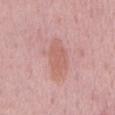<record>
  <biopsy_status>not biopsied; imaged during a skin examination</biopsy_status>
  <patient>
    <sex>male</sex>
    <age_approx>60</age_approx>
  </patient>
  <image>
    <source>total-body photography crop</source>
    <field_of_view_mm>15</field_of_view_mm>
  </image>
  <site>back</site>
  <lesion_size>
    <long_diameter_mm_approx>5.0</long_diameter_mm_approx>
  </lesion_size>
  <lighting>white-light</lighting>
</record>